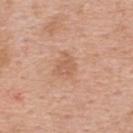Findings:
• workup — total-body-photography surveillance lesion; no biopsy
• image source — ~15 mm crop, total-body skin-cancer survey
• location — the upper back
• patient — female, aged 38–42